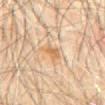Assessment:
This lesion was catalogued during total-body skin photography and was not selected for biopsy.
Context:
Captured under cross-polarized illumination. The subject is a male aged approximately 60. Measured at roughly 2.5 mm in maximum diameter. A 15 mm close-up extracted from a 3D total-body photography capture. Located on the mid back.